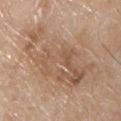The lesion is on the chest. A male subject aged 78 to 82. Captured under white-light illumination. Approximately 12.5 mm at its widest. A 15 mm close-up extracted from a 3D total-body photography capture.